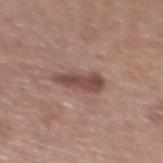| field | value |
|---|---|
| biopsy status | imaged on a skin check; not biopsied |
| patient | female, approximately 75 years of age |
| acquisition | ~15 mm tile from a whole-body skin photo |
| anatomic site | the back |